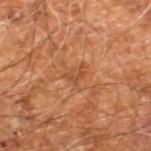Clinical impression: Part of a total-body skin-imaging series; this lesion was reviewed on a skin check and was not flagged for biopsy. Clinical summary: The tile uses cross-polarized illumination. The lesion is located on the right leg. Automated image analysis of the tile measured a footprint of about 3 mm², an outline eccentricity of about 0.75 (0 = round, 1 = elongated), and a shape-asymmetry score of about 0.5 (0 = symmetric). It also reported a border-irregularity rating of about 7/10. Measured at roughly 2.5 mm in maximum diameter. A lesion tile, about 15 mm wide, cut from a 3D total-body photograph. A male patient aged 58 to 62.